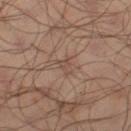  image:
    source: total-body photography crop
    field_of_view_mm: 15
  automated_metrics:
    area_mm2_approx: 3.5
    border_irregularity_0_10: 5.5
    color_variation_0_10: 1.0
  site: left thigh
  lighting: cross-polarized
  patient:
    sex: male
    age_approx: 65
  lesion_size:
    long_diameter_mm_approx: 2.5